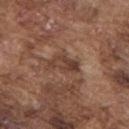Q: Was a biopsy performed?
A: catalogued during a skin exam; not biopsied
Q: What is the anatomic site?
A: the upper back
Q: How was the tile lit?
A: white-light
Q: Patient demographics?
A: male, aged approximately 75
Q: What is the imaging modality?
A: ~15 mm tile from a whole-body skin photo
Q: Lesion size?
A: ~4 mm (longest diameter)